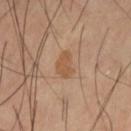workup=catalogued during a skin exam; not biopsied.